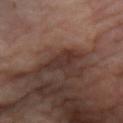Captured during whole-body skin photography for melanoma surveillance; the lesion was not biopsied.
A 15 mm close-up extracted from a 3D total-body photography capture.
Automated image analysis of the tile measured border irregularity of about 2.5 on a 0–10 scale, a within-lesion color-variation index near 3/10, and radial color variation of about 1.
Imaged with cross-polarized lighting.
The lesion is located on the chest.
About 3.5 mm across.
A female subject aged around 50.